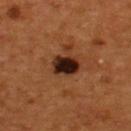This lesion was catalogued during total-body skin photography and was not selected for biopsy.
The lesion is on the upper back.
A lesion tile, about 15 mm wide, cut from a 3D total-body photograph.
An algorithmic analysis of the crop reported an area of roughly 6.5 mm² and an outline eccentricity of about 0.7 (0 = round, 1 = elongated). And it measured a lesion color around L≈21 a*≈19 b*≈23 in CIELAB, about 17 CIELAB-L* units darker than the surrounding skin, and a lesion-to-skin contrast of about 18.5 (normalized; higher = more distinct). The analysis additionally found a border-irregularity rating of about 1.5/10, a color-variation rating of about 5.5/10, and peripheral color asymmetry of about 1. The analysis additionally found an automated nevus-likeness rating near 85 out of 100 and a detector confidence of about 100 out of 100 that the crop contains a lesion.
Measured at roughly 3 mm in maximum diameter.
The subject is a male aged 53–57.
Captured under cross-polarized illumination.